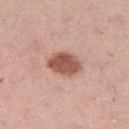Captured during whole-body skin photography for melanoma surveillance; the lesion was not biopsied. The lesion is on the leg. Imaged with white-light lighting. This image is a 15 mm lesion crop taken from a total-body photograph. The recorded lesion diameter is about 4.5 mm. A female subject aged around 40. An algorithmic analysis of the crop reported an area of roughly 9.5 mm² and a shape eccentricity near 0.7. It also reported a border-irregularity index near 1/10, a color-variation rating of about 3.5/10, and radial color variation of about 1. And it measured a nevus-likeness score of about 95/100.